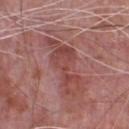Findings:
* follow-up — catalogued during a skin exam; not biopsied
* illumination — white-light
* anatomic site — the front of the torso
* automated lesion analysis — a lesion area of about 13 mm², a shape eccentricity near 0.95, and a symmetry-axis asymmetry near 0.5; an average lesion color of about L≈45 a*≈26 b*≈24 (CIELAB), roughly 8 lightness units darker than nearby skin, and a normalized border contrast of about 6.5; border irregularity of about 8.5 on a 0–10 scale, internal color variation of about 3.5 on a 0–10 scale, and a peripheral color-asymmetry measure near 1; a nevus-likeness score of about 0/100 and a detector confidence of about 85 out of 100 that the crop contains a lesion
* lesion diameter — about 7 mm
* imaging modality — ~15 mm tile from a whole-body skin photo
* subject — male, aged approximately 70